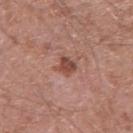workup: total-body-photography surveillance lesion; no biopsy
automated metrics: an outline eccentricity of about 0.65 (0 = round, 1 = elongated) and two-axis asymmetry of about 0.25; a lesion color around L≈47 a*≈23 b*≈27 in CIELAB, roughly 11 lightness units darker than nearby skin, and a lesion-to-skin contrast of about 8.5 (normalized; higher = more distinct); a border-irregularity rating of about 2.5/10 and a color-variation rating of about 2/10; a classifier nevus-likeness of about 60/100 and lesion-presence confidence of about 100/100
body site: the left upper arm
tile lighting: white-light illumination
subject: male, about 80 years old
image: total-body-photography crop, ~15 mm field of view
lesion size: about 3 mm